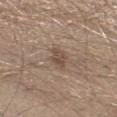Impression:
Imaged during a routine full-body skin examination; the lesion was not biopsied and no histopathology is available.
Background:
A 15 mm close-up tile from a total-body photography series done for melanoma screening. A male patient, aged 28–32. The lesion is on the left lower leg.